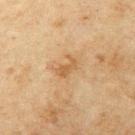Recorded during total-body skin imaging; not selected for excision or biopsy. The lesion is located on the left upper arm. A close-up tile cropped from a whole-body skin photograph, about 15 mm across. A male subject, approximately 65 years of age. Imaged with cross-polarized lighting.